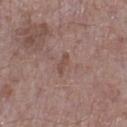* biopsy status — catalogued during a skin exam; not biopsied
* patient — male, aged 68–72
* lesion diameter — about 2.5 mm
* tile lighting — white-light
* body site — the right lower leg
* image source — total-body-photography crop, ~15 mm field of view